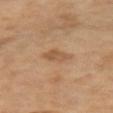Assessment:
Recorded during total-body skin imaging; not selected for excision or biopsy.
Background:
The tile uses cross-polarized illumination. Approximately 3.5 mm at its widest. A male subject, aged 63–67. A roughly 15 mm field-of-view crop from a total-body skin photograph. The lesion is on the left upper arm.